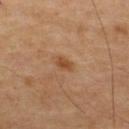notes: imaged on a skin check; not biopsied | image: total-body-photography crop, ~15 mm field of view | location: the upper back | diameter: ≈2.5 mm | image-analysis metrics: a symmetry-axis asymmetry near 0.35; border irregularity of about 3 on a 0–10 scale, a color-variation rating of about 1.5/10, and peripheral color asymmetry of about 0.5; a nevus-likeness score of about 65/100 and a detector confidence of about 100 out of 100 that the crop contains a lesion | patient: male, aged 53–57.